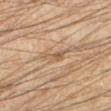Q: Is there a histopathology result?
A: imaged on a skin check; not biopsied
Q: What is the imaging modality?
A: total-body-photography crop, ~15 mm field of view
Q: How was the tile lit?
A: cross-polarized illumination
Q: Automated lesion metrics?
A: a mean CIELAB color near L≈56 a*≈17 b*≈32, roughly 8 lightness units darker than nearby skin, and a normalized lesion–skin contrast near 5.5; a color-variation rating of about 2.5/10 and peripheral color asymmetry of about 1; a nevus-likeness score of about 0/100
Q: Who is the patient?
A: male, aged 43–47
Q: Lesion location?
A: the left lower leg